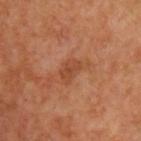Impression:
The lesion was tiled from a total-body skin photograph and was not biopsied.
Acquisition and patient details:
A male patient aged around 65. A roughly 15 mm field-of-view crop from a total-body skin photograph. On the chest.